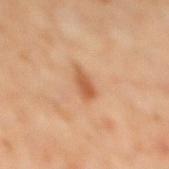biopsy status: no biopsy performed (imaged during a skin exam); site: the mid back; patient: male, in their 60s; lesion diameter: about 3 mm; acquisition: ~15 mm tile from a whole-body skin photo.